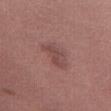The lesion was tiled from a total-body skin photograph and was not biopsied. A lesion tile, about 15 mm wide, cut from a 3D total-body photograph. A female patient aged approximately 60. This is a white-light tile. Measured at roughly 4 mm in maximum diameter. The total-body-photography lesion software estimated a mean CIELAB color near L≈46 a*≈21 b*≈21 and a normalized lesion–skin contrast near 6. It also reported border irregularity of about 4 on a 0–10 scale, a within-lesion color-variation index near 2/10, and a peripheral color-asymmetry measure near 0.5. It also reported lesion-presence confidence of about 100/100. On the abdomen.